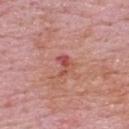Part of a total-body skin-imaging series; this lesion was reviewed on a skin check and was not flagged for biopsy. Imaged with white-light lighting. The subject is a male aged 63–67. A roughly 15 mm field-of-view crop from a total-body skin photograph. Located on the upper back.